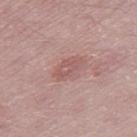The lesion was tiled from a total-body skin photograph and was not biopsied.
A close-up tile cropped from a whole-body skin photograph, about 15 mm across.
The lesion-visualizer software estimated a detector confidence of about 100 out of 100 that the crop contains a lesion.
Longest diameter approximately 3.5 mm.
A male patient roughly 50 years of age.
Located on the right thigh.